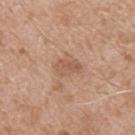Q: Was this lesion biopsied?
A: total-body-photography surveillance lesion; no biopsy
Q: What is the imaging modality?
A: ~15 mm crop, total-body skin-cancer survey
Q: What lighting was used for the tile?
A: white-light illumination
Q: Where on the body is the lesion?
A: the upper back
Q: Who is the patient?
A: male, approximately 65 years of age
Q: What is the lesion's diameter?
A: ≈3 mm
Q: Automated lesion metrics?
A: an automated nevus-likeness rating near 0 out of 100 and lesion-presence confidence of about 100/100